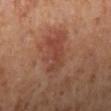<lesion>
  <biopsy_status>not biopsied; imaged during a skin examination</biopsy_status>
  <automated_metrics>
    <area_mm2_approx>7.0</area_mm2_approx>
    <eccentricity>0.9</eccentricity>
    <cielab_L>43</cielab_L>
    <cielab_a>25</cielab_a>
    <cielab_b>29</cielab_b>
    <vs_skin_darker_L>7.0</vs_skin_darker_L>
    <vs_skin_contrast_norm>5.5</vs_skin_contrast_norm>
    <border_irregularity_0_10>6.0</border_irregularity_0_10>
    <color_variation_0_10>2.0</color_variation_0_10>
    <peripheral_color_asymmetry>0.5</peripheral_color_asymmetry>
  </automated_metrics>
  <patient>
    <sex>female</sex>
    <age_approx>55</age_approx>
  </patient>
  <lesion_size>
    <long_diameter_mm_approx>4.0</long_diameter_mm_approx>
  </lesion_size>
  <site>leg</site>
  <lighting>cross-polarized</lighting>
  <image>
    <source>total-body photography crop</source>
    <field_of_view_mm>15</field_of_view_mm>
  </image>
</lesion>